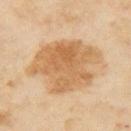Q: Was a biopsy performed?
A: imaged on a skin check; not biopsied
Q: What is the anatomic site?
A: the right upper arm
Q: How was the tile lit?
A: cross-polarized
Q: What did automated image analysis measure?
A: a footprint of about 35 mm², a shape eccentricity near 0.6, and two-axis asymmetry of about 0.3; a mean CIELAB color near L≈61 a*≈18 b*≈38; a detector confidence of about 100 out of 100 that the crop contains a lesion
Q: How large is the lesion?
A: ~8.5 mm (longest diameter)
Q: Who is the patient?
A: female, in their mid-30s
Q: How was this image acquired?
A: ~15 mm tile from a whole-body skin photo